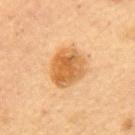| field | value |
|---|---|
| notes | imaged on a skin check; not biopsied |
| subject | female, aged approximately 60 |
| diameter | ≈5 mm |
| image source | ~15 mm tile from a whole-body skin photo |
| lighting | cross-polarized |
| location | the mid back |